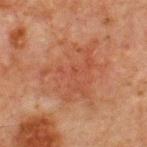Background:
The lesion's longest dimension is about 7.5 mm. The lesion is located on the chest. Imaged with cross-polarized lighting. The lesion-visualizer software estimated an area of roughly 31 mm², an eccentricity of roughly 0.5, and a symmetry-axis asymmetry near 0.35. The analysis additionally found an automated nevus-likeness rating near 0 out of 100 and lesion-presence confidence of about 100/100. A male subject roughly 65 years of age. Cropped from a total-body skin-imaging series; the visible field is about 15 mm.